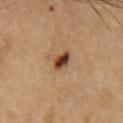Captured during whole-body skin photography for melanoma surveillance; the lesion was not biopsied. Longest diameter approximately 3 mm. A male subject roughly 70 years of age. From the chest. This image is a 15 mm lesion crop taken from a total-body photograph. Automated image analysis of the tile measured internal color variation of about 6 on a 0–10 scale. The tile uses cross-polarized illumination.